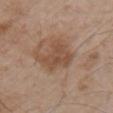No biopsy was performed on this lesion — it was imaged during a full skin examination and was not determined to be concerning. Imaged with white-light lighting. The lesion's longest dimension is about 4 mm. An algorithmic analysis of the crop reported an average lesion color of about L≈49 a*≈19 b*≈29 (CIELAB), roughly 8 lightness units darker than nearby skin, and a lesion-to-skin contrast of about 6.5 (normalized; higher = more distinct). The lesion is on the right upper arm. Cropped from a total-body skin-imaging series; the visible field is about 15 mm. A male patient, in their mid- to late 50s.